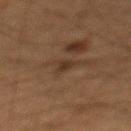Part of a total-body skin-imaging series; this lesion was reviewed on a skin check and was not flagged for biopsy. Automated image analysis of the tile measured a footprint of about 4 mm² and a shape-asymmetry score of about 0.5 (0 = symmetric). The software also gave a mean CIELAB color near L≈28 a*≈13 b*≈23, roughly 6 lightness units darker than nearby skin, and a lesion-to-skin contrast of about 6.5 (normalized; higher = more distinct). And it measured border irregularity of about 4.5 on a 0–10 scale, a color-variation rating of about 1.5/10, and radial color variation of about 0.5. Cropped from a whole-body photographic skin survey; the tile spans about 15 mm. A male patient, about 65 years old. On the mid back. Imaged with cross-polarized lighting. Longest diameter approximately 3 mm.